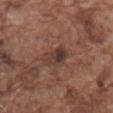Assessment:
Part of a total-body skin-imaging series; this lesion was reviewed on a skin check and was not flagged for biopsy.
Acquisition and patient details:
A 15 mm close-up tile from a total-body photography series done for melanoma screening. The subject is a male aged approximately 75. Imaged with white-light lighting. Located on the mid back. Approximately 3.5 mm at its widest.